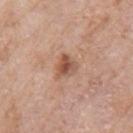• follow-up: imaged on a skin check; not biopsied
• anatomic site: the left upper arm
• image: ~15 mm tile from a whole-body skin photo
• patient: female, aged approximately 70